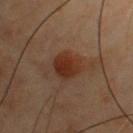biopsy status: imaged on a skin check; not biopsied | lesion size: ≈3.5 mm | automated lesion analysis: a mean CIELAB color near L≈24 a*≈18 b*≈24, about 8 CIELAB-L* units darker than the surrounding skin, and a normalized border contrast of about 9.5; border irregularity of about 1.5 on a 0–10 scale, a color-variation rating of about 3/10, and a peripheral color-asymmetry measure near 1 | acquisition: ~15 mm tile from a whole-body skin photo | lighting: cross-polarized illumination | location: the chest | patient: male, aged around 55.